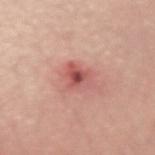biopsy_status: not biopsied; imaged during a skin examination